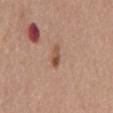Findings:
• biopsy status — catalogued during a skin exam; not biopsied
• body site — the mid back
• image source — ~15 mm tile from a whole-body skin photo
• patient — male, aged around 65